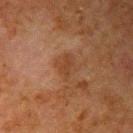site: the arm | image: ~15 mm crop, total-body skin-cancer survey | patient: male, roughly 80 years of age.